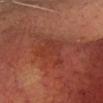Assessment: The lesion was photographed on a routine skin check and not biopsied; there is no pathology result. Context: Measured at roughly 5.5 mm in maximum diameter. A 15 mm close-up tile from a total-body photography series done for melanoma screening. Imaged with cross-polarized lighting. An algorithmic analysis of the crop reported a lesion color around L≈33 a*≈26 b*≈28 in CIELAB. The analysis additionally found a nevus-likeness score of about 25/100 and a detector confidence of about 85 out of 100 that the crop contains a lesion. On the head or neck. A male subject aged 58–62.